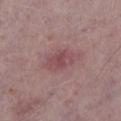biopsy status: catalogued during a skin exam; not biopsied
TBP lesion metrics: an area of roughly 6.5 mm², an eccentricity of roughly 0.75, and a symmetry-axis asymmetry near 0.2; a lesion color around L≈47 a*≈24 b*≈17 in CIELAB, a lesion–skin lightness drop of about 8, and a normalized lesion–skin contrast near 6; border irregularity of about 2 on a 0–10 scale and a peripheral color-asymmetry measure near 1; a classifier nevus-likeness of about 0/100
lighting: white-light
image source: total-body-photography crop, ~15 mm field of view
site: the left lower leg
lesion diameter: about 3.5 mm
patient: male, roughly 75 years of age